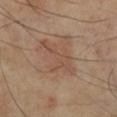{
  "patient": {
    "sex": "female",
    "age_approx": 70
  },
  "lesion_size": {
    "long_diameter_mm_approx": 5.0
  },
  "lighting": "cross-polarized",
  "image": {
    "source": "total-body photography crop",
    "field_of_view_mm": 15
  },
  "site": "left lower leg"
}